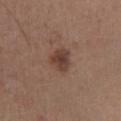This lesion was catalogued during total-body skin photography and was not selected for biopsy. This is a white-light tile. Cropped from a whole-body photographic skin survey; the tile spans about 15 mm. On the right lower leg. The patient is a male aged 58 to 62. About 3 mm across.